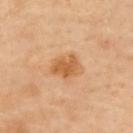notes = imaged on a skin check; not biopsied | image source = ~15 mm crop, total-body skin-cancer survey | automated metrics = a border-irregularity index near 2.5/10, a color-variation rating of about 3/10, and radial color variation of about 1; a classifier nevus-likeness of about 80/100 and lesion-presence confidence of about 100/100 | lesion diameter = about 3.5 mm | patient = male, aged around 65 | site = the back.